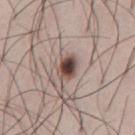Q: What lighting was used for the tile?
A: white-light illumination
Q: How was this image acquired?
A: ~15 mm crop, total-body skin-cancer survey
Q: How large is the lesion?
A: ~3 mm (longest diameter)
Q: Automated lesion metrics?
A: a footprint of about 5.5 mm², an outline eccentricity of about 0.6 (0 = round, 1 = elongated), and two-axis asymmetry of about 0.15; a border-irregularity rating of about 1.5/10, a within-lesion color-variation index near 9.5/10, and a peripheral color-asymmetry measure near 3
Q: Where on the body is the lesion?
A: the abdomen
Q: What are the patient's age and sex?
A: male, about 35 years old